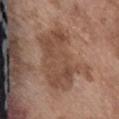Impression:
This lesion was catalogued during total-body skin photography and was not selected for biopsy.
Acquisition and patient details:
From the abdomen. A male patient approximately 75 years of age. A lesion tile, about 15 mm wide, cut from a 3D total-body photograph.